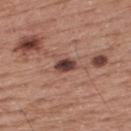Assessment: Imaged during a routine full-body skin examination; the lesion was not biopsied and no histopathology is available. Context: Longest diameter approximately 2.5 mm. A male subject aged 53 to 57. An algorithmic analysis of the crop reported a border-irregularity index near 2/10 and radial color variation of about 1.5. The lesion is located on the upper back. A 15 mm crop from a total-body photograph taken for skin-cancer surveillance.